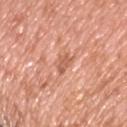Case summary:
* image source · 15 mm crop, total-body photography
* subject · male, aged 43–47
* site · the upper back
* diameter · ≈3 mm